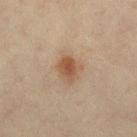Q: Was a biopsy performed?
A: catalogued during a skin exam; not biopsied
Q: What kind of image is this?
A: 15 mm crop, total-body photography
Q: How large is the lesion?
A: ≈3.5 mm
Q: What did automated image analysis measure?
A: a border-irregularity rating of about 2/10, internal color variation of about 4 on a 0–10 scale, and radial color variation of about 1
Q: Who is the patient?
A: female, in their mid- to late 40s
Q: Where on the body is the lesion?
A: the left lower leg
Q: Illumination type?
A: cross-polarized illumination